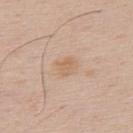workup — total-body-photography surveillance lesion; no biopsy
patient — male, aged 53–57
image source — 15 mm crop, total-body photography
anatomic site — the upper back